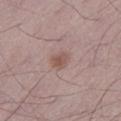Recorded during total-body skin imaging; not selected for excision or biopsy.
The lesion is located on the left lower leg.
This image is a 15 mm lesion crop taken from a total-body photograph.
A male subject aged 48–52.